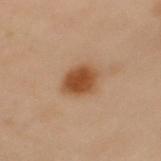The lesion was photographed on a routine skin check and not biopsied; there is no pathology result. Located on the upper back. A region of skin cropped from a whole-body photographic capture, roughly 15 mm wide. The lesion-visualizer software estimated an eccentricity of roughly 0.7. The software also gave a nevus-likeness score of about 100/100 and a detector confidence of about 100 out of 100 that the crop contains a lesion. A female patient, approximately 60 years of age.